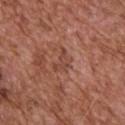Q: Was a biopsy performed?
A: catalogued during a skin exam; not biopsied
Q: Illumination type?
A: white-light
Q: What is the anatomic site?
A: the upper back
Q: Patient demographics?
A: male, in their mid-70s
Q: What kind of image is this?
A: total-body-photography crop, ~15 mm field of view
Q: How large is the lesion?
A: about 3 mm